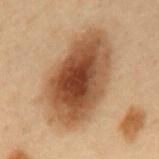Imaged during a routine full-body skin examination; the lesion was not biopsied and no histopathology is available.
Imaged with cross-polarized lighting.
The lesion-visualizer software estimated a shape eccentricity near 0.85 and a shape-asymmetry score of about 0.15 (0 = symmetric). It also reported a lesion color around L≈40 a*≈17 b*≈29 in CIELAB, about 14 CIELAB-L* units darker than the surrounding skin, and a lesion-to-skin contrast of about 11 (normalized; higher = more distinct). It also reported a within-lesion color-variation index near 7.5/10 and a peripheral color-asymmetry measure near 2. The software also gave a classifier nevus-likeness of about 100/100 and a lesion-detection confidence of about 100/100.
The lesion is located on the mid back.
A male patient approximately 55 years of age.
A close-up tile cropped from a whole-body skin photograph, about 15 mm across.